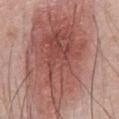Assessment:
Imaged during a routine full-body skin examination; the lesion was not biopsied and no histopathology is available.
Context:
On the front of the torso. The lesion's longest dimension is about 18.5 mm. The tile uses white-light illumination. A male patient, aged around 70. A 15 mm close-up extracted from a 3D total-body photography capture. The lesion-visualizer software estimated a footprint of about 125 mm², a shape eccentricity near 0.8, and two-axis asymmetry of about 0.25. And it measured a mean CIELAB color near L≈54 a*≈23 b*≈24 and a normalized border contrast of about 8. The software also gave internal color variation of about 8 on a 0–10 scale and a peripheral color-asymmetry measure near 2.5. And it measured a nevus-likeness score of about 100/100 and a lesion-detection confidence of about 100/100.